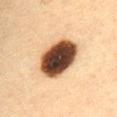The tile uses cross-polarized illumination.
A female patient aged 58–62.
A lesion tile, about 15 mm wide, cut from a 3D total-body photograph.
The lesion-visualizer software estimated an average lesion color of about L≈38 a*≈17 b*≈27 (CIELAB), roughly 27 lightness units darker than nearby skin, and a lesion-to-skin contrast of about 19 (normalized; higher = more distinct). The software also gave a border-irregularity index near 1.5/10 and a peripheral color-asymmetry measure near 2.5.
Approximately 6.5 mm at its widest.
The lesion is located on the abdomen.